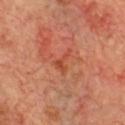No biopsy was performed on this lesion — it was imaged during a full skin examination and was not determined to be concerning.
A close-up tile cropped from a whole-body skin photograph, about 15 mm across.
On the chest.
Imaged with cross-polarized lighting.
Automated image analysis of the tile measured an area of roughly 3 mm², a shape eccentricity near 0.8, and a shape-asymmetry score of about 0.7 (0 = symmetric). It also reported a border-irregularity rating of about 7.5/10, a within-lesion color-variation index near 0/10, and peripheral color asymmetry of about 0. The analysis additionally found a nevus-likeness score of about 0/100.
A male patient approximately 70 years of age.
Approximately 3 mm at its widest.